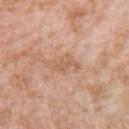Recorded during total-body skin imaging; not selected for excision or biopsy.
The lesion is located on the right upper arm.
A region of skin cropped from a whole-body photographic capture, roughly 15 mm wide.
Measured at roughly 4 mm in maximum diameter.
Automated image analysis of the tile measured a footprint of about 5 mm² and a shape-asymmetry score of about 0.4 (0 = symmetric). And it measured an average lesion color of about L≈60 a*≈20 b*≈33 (CIELAB), about 8 CIELAB-L* units darker than the surrounding skin, and a lesion-to-skin contrast of about 5.5 (normalized; higher = more distinct). The analysis additionally found a border-irregularity rating of about 5.5/10 and peripheral color asymmetry of about 0.5. The software also gave a nevus-likeness score of about 0/100 and a detector confidence of about 100 out of 100 that the crop contains a lesion.
A female patient, aged approximately 40.